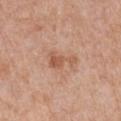Clinical impression: The lesion was tiled from a total-body skin photograph and was not biopsied. Clinical summary: About 3.5 mm across. A male subject, roughly 55 years of age. The tile uses white-light illumination. A region of skin cropped from a whole-body photographic capture, roughly 15 mm wide. The lesion is on the left upper arm.